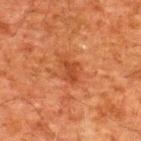Clinical impression: The lesion was photographed on a routine skin check and not biopsied; there is no pathology result.